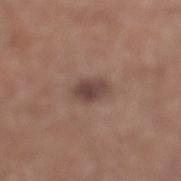Clinical summary: Located on the leg. About 3 mm across. The subject is a male approximately 65 years of age. A lesion tile, about 15 mm wide, cut from a 3D total-body photograph.